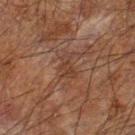{
  "biopsy_status": "not biopsied; imaged during a skin examination",
  "site": "left forearm",
  "image": {
    "source": "total-body photography crop",
    "field_of_view_mm": 15
  },
  "lesion_size": {
    "long_diameter_mm_approx": 3.0
  },
  "patient": {
    "sex": "male",
    "age_approx": 65
  },
  "automated_metrics": {
    "vs_skin_darker_L": 5.0,
    "border_irregularity_0_10": 5.5,
    "peripheral_color_asymmetry": 0.0,
    "lesion_detection_confidence_0_100": 90
  },
  "lighting": "cross-polarized"
}